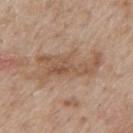Recorded during total-body skin imaging; not selected for excision or biopsy.